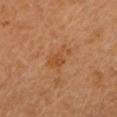Clinical impression:
The lesion was tiled from a total-body skin photograph and was not biopsied.
Acquisition and patient details:
A female patient aged around 65. Located on the head or neck. A 15 mm close-up extracted from a 3D total-body photography capture. The lesion's longest dimension is about 4 mm.